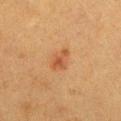Impression:
Imaged during a routine full-body skin examination; the lesion was not biopsied and no histopathology is available.
Acquisition and patient details:
A female subject aged 38 to 42. The recorded lesion diameter is about 3 mm. This image is a 15 mm lesion crop taken from a total-body photograph. Captured under cross-polarized illumination. Automated image analysis of the tile measured a mean CIELAB color near L≈44 a*≈21 b*≈33, roughly 7 lightness units darker than nearby skin, and a lesion-to-skin contrast of about 6 (normalized; higher = more distinct). It also reported a border-irregularity index near 2/10, a color-variation rating of about 4/10, and radial color variation of about 1.5. The lesion is located on the chest.